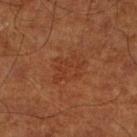workup = no biopsy performed (imaged during a skin exam)
tile lighting = cross-polarized illumination
acquisition = ~15 mm crop, total-body skin-cancer survey
diameter = ≈4.5 mm
patient = about 65 years old
location = the leg